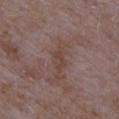Clinical impression: This lesion was catalogued during total-body skin photography and was not selected for biopsy. Clinical summary: The subject is a male roughly 65 years of age. The lesion-visualizer software estimated internal color variation of about 3 on a 0–10 scale and peripheral color asymmetry of about 1. The tile uses white-light illumination. A region of skin cropped from a whole-body photographic capture, roughly 15 mm wide. The lesion is located on the left upper arm.